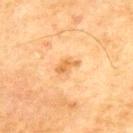Q: Was a biopsy performed?
A: no biopsy performed (imaged during a skin exam)
Q: What kind of image is this?
A: 15 mm crop, total-body photography
Q: Lesion location?
A: the upper back
Q: Illumination type?
A: cross-polarized
Q: Who is the patient?
A: male, about 70 years old
Q: How large is the lesion?
A: about 3 mm
Q: Automated lesion metrics?
A: an average lesion color of about L≈56 a*≈21 b*≈41 (CIELAB), roughly 9 lightness units darker than nearby skin, and a lesion-to-skin contrast of about 7 (normalized; higher = more distinct); a classifier nevus-likeness of about 10/100